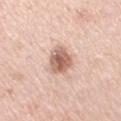Assessment:
No biopsy was performed on this lesion — it was imaged during a full skin examination and was not determined to be concerning.
Acquisition and patient details:
On the arm. This image is a 15 mm lesion crop taken from a total-body photograph. The subject is a female aged approximately 40. Imaged with white-light lighting.